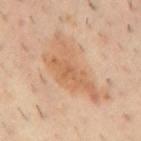Findings:
* follow-up: imaged on a skin check; not biopsied
* image source: ~15 mm crop, total-body skin-cancer survey
* illumination: cross-polarized illumination
* diameter: ≈9 mm
* automated lesion analysis: an outline eccentricity of about 0.9 (0 = round, 1 = elongated) and a symmetry-axis asymmetry near 0.5; an average lesion color of about L≈61 a*≈20 b*≈35 (CIELAB), a lesion–skin lightness drop of about 9, and a normalized lesion–skin contrast near 6.5; a color-variation rating of about 3.5/10; a nevus-likeness score of about 5/100
* body site: the mid back
* subject: male, approximately 40 years of age